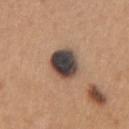Recorded during total-body skin imaging; not selected for excision or biopsy.
The recorded lesion diameter is about 4 mm.
A region of skin cropped from a whole-body photographic capture, roughly 15 mm wide.
A male subject, aged 63–67.
On the chest.